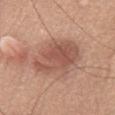<case>
<biopsy_status>not biopsied; imaged during a skin examination</biopsy_status>
<image>
  <source>total-body photography crop</source>
  <field_of_view_mm>15</field_of_view_mm>
</image>
<patient>
  <sex>male</sex>
  <age_approx>75</age_approx>
</patient>
<lighting>white-light</lighting>
<automated_metrics>
  <cielab_L>54</cielab_L>
  <cielab_a>22</cielab_a>
  <cielab_b>28</cielab_b>
  <vs_skin_contrast_norm>7.0</vs_skin_contrast_norm>
  <lesion_detection_confidence_0_100>100</lesion_detection_confidence_0_100>
</automated_metrics>
<lesion_size>
  <long_diameter_mm_approx>6.0</long_diameter_mm_approx>
</lesion_size>
<site>chest</site>
</case>